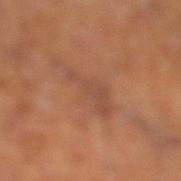<tbp_lesion>
  <biopsy_status>not biopsied; imaged during a skin examination</biopsy_status>
  <patient>
    <sex>male</sex>
    <age_approx>60</age_approx>
  </patient>
  <automated_metrics>
    <vs_skin_contrast_norm>5.0</vs_skin_contrast_norm>
    <nevus_likeness_0_100>0</nevus_likeness_0_100>
    <lesion_detection_confidence_0_100>100</lesion_detection_confidence_0_100>
  </automated_metrics>
  <image>
    <source>total-body photography crop</source>
    <field_of_view_mm>15</field_of_view_mm>
  </image>
  <lighting>cross-polarized</lighting>
  <lesion_size>
    <long_diameter_mm_approx>6.5</long_diameter_mm_approx>
  </lesion_size>
  <site>right lower leg</site>
</tbp_lesion>